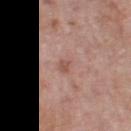{
  "biopsy_status": "not biopsied; imaged during a skin examination",
  "site": "left thigh",
  "lighting": "white-light",
  "patient": {
    "sex": "female",
    "age_approx": 50
  },
  "image": {
    "source": "total-body photography crop",
    "field_of_view_mm": 15
  },
  "automated_metrics": {
    "shape_asymmetry": 0.25,
    "cielab_L": 56,
    "cielab_a": 20,
    "cielab_b": 25,
    "vs_skin_darker_L": 6.0,
    "vs_skin_contrast_norm": 4.5
  }
}